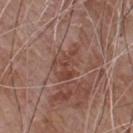This lesion was catalogued during total-body skin photography and was not selected for biopsy.
The lesion is located on the front of the torso.
The total-body-photography lesion software estimated an average lesion color of about L≈43 a*≈22 b*≈25 (CIELAB) and about 7 CIELAB-L* units darker than the surrounding skin. The software also gave a classifier nevus-likeness of about 0/100.
Cropped from a total-body skin-imaging series; the visible field is about 15 mm.
The recorded lesion diameter is about 3.5 mm.
A male patient, aged approximately 80.